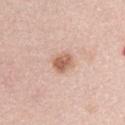Captured during whole-body skin photography for melanoma surveillance; the lesion was not biopsied. A lesion tile, about 15 mm wide, cut from a 3D total-body photograph. The lesion is located on the abdomen. The total-body-photography lesion software estimated an area of roughly 5.5 mm², a shape eccentricity near 0.55, and a symmetry-axis asymmetry near 0.15. The software also gave a lesion color around L≈61 a*≈21 b*≈30 in CIELAB and a lesion-to-skin contrast of about 8 (normalized; higher = more distinct). It also reported lesion-presence confidence of about 100/100. A female patient, in their mid-60s.